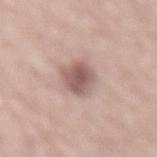Captured during whole-body skin photography for melanoma surveillance; the lesion was not biopsied.
Automated tile analysis of the lesion measured a lesion area of about 9 mm², an outline eccentricity of about 0.75 (0 = round, 1 = elongated), and two-axis asymmetry of about 0.2. It also reported a border-irregularity index near 2/10, internal color variation of about 5 on a 0–10 scale, and a peripheral color-asymmetry measure near 1.5. The analysis additionally found an automated nevus-likeness rating near 60 out of 100 and lesion-presence confidence of about 100/100.
A male subject aged approximately 60.
A region of skin cropped from a whole-body photographic capture, roughly 15 mm wide.
The tile uses white-light illumination.
Approximately 4.5 mm at its widest.
On the lower back.